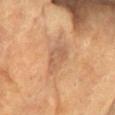subject=female, aged around 80 | illumination=cross-polarized illumination | location=the chest | image=total-body-photography crop, ~15 mm field of view | size=~4 mm (longest diameter).